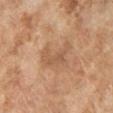{
  "biopsy_status": "not biopsied; imaged during a skin examination",
  "lighting": "cross-polarized",
  "site": "left lower leg",
  "lesion_size": {
    "long_diameter_mm_approx": 4.0
  },
  "image": {
    "source": "total-body photography crop",
    "field_of_view_mm": 15
  },
  "patient": {
    "sex": "female",
    "age_approx": 60
  },
  "automated_metrics": {
    "area_mm2_approx": 8.5,
    "eccentricity": 0.8,
    "shape_asymmetry": 0.45
  }
}